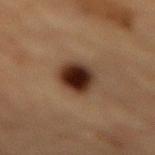{"biopsy_status": "not biopsied; imaged during a skin examination", "patient": {"sex": "male", "age_approx": "85+"}, "site": "mid back", "lesion_size": {"long_diameter_mm_approx": 4.0}, "image": {"source": "total-body photography crop", "field_of_view_mm": 15}, "lighting": "cross-polarized"}